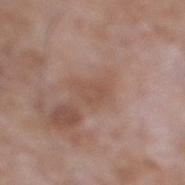Imaged during a routine full-body skin examination; the lesion was not biopsied and no histopathology is available.
A male subject aged approximately 60.
A roughly 15 mm field-of-view crop from a total-body skin photograph.
The lesion's longest dimension is about 3 mm.
The lesion is on the right lower leg.